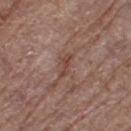Impression: This lesion was catalogued during total-body skin photography and was not selected for biopsy. Image and clinical context: Longest diameter approximately 3 mm. A female subject, aged around 80. A roughly 15 mm field-of-view crop from a total-body skin photograph. Located on the left thigh.